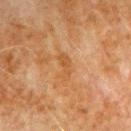Assessment: The lesion was photographed on a routine skin check and not biopsied; there is no pathology result. Image and clinical context: About 3.5 mm across. From the left upper arm. A male patient aged around 80. A region of skin cropped from a whole-body photographic capture, roughly 15 mm wide. Captured under cross-polarized illumination.